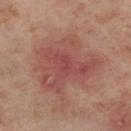Notes:
- follow-up · catalogued during a skin exam; not biopsied
- imaging modality · ~15 mm tile from a whole-body skin photo
- diameter · about 7.5 mm
- subject · female, aged approximately 55
- body site · the right thigh